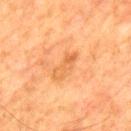Clinical impression: Recorded during total-body skin imaging; not selected for excision or biopsy. Background: A male subject aged 78–82. A close-up tile cropped from a whole-body skin photograph, about 15 mm across. The lesion is located on the upper back.